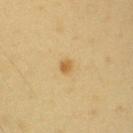<lesion>
  <biopsy_status>not biopsied; imaged during a skin examination</biopsy_status>
  <lesion_size>
    <long_diameter_mm_approx>1.5</long_diameter_mm_approx>
  </lesion_size>
  <site>left upper arm</site>
  <image>
    <source>total-body photography crop</source>
    <field_of_view_mm>15</field_of_view_mm>
  </image>
  <patient>
    <sex>male</sex>
    <age_approx>65</age_approx>
  </patient>
  <lighting>cross-polarized</lighting>
</lesion>